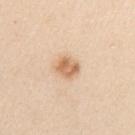patient = male, aged 58 to 62; tile lighting = white-light; site = the arm; lesion diameter = ≈3 mm; image source = ~15 mm tile from a whole-body skin photo.